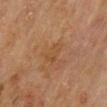Recorded during total-body skin imaging; not selected for excision or biopsy.
The lesion's longest dimension is about 3 mm.
The patient is a male roughly 70 years of age.
Captured under cross-polarized illumination.
On the mid back.
A close-up tile cropped from a whole-body skin photograph, about 15 mm across.